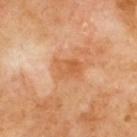Impression: This lesion was catalogued during total-body skin photography and was not selected for biopsy. Acquisition and patient details: From the upper back. About 3.5 mm across. This is a cross-polarized tile. The lesion-visualizer software estimated a footprint of about 7 mm², an eccentricity of roughly 0.7, and two-axis asymmetry of about 0.25. It also reported a mean CIELAB color near L≈58 a*≈25 b*≈40, about 8 CIELAB-L* units darker than the surrounding skin, and a normalized lesion–skin contrast near 6. This image is a 15 mm lesion crop taken from a total-body photograph. The subject is a male aged around 70.